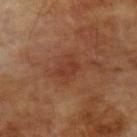{
  "biopsy_status": "not biopsied; imaged during a skin examination",
  "patient": {
    "sex": "male",
    "age_approx": 70
  },
  "site": "left upper arm",
  "image": {
    "source": "total-body photography crop",
    "field_of_view_mm": 15
  },
  "lighting": "cross-polarized",
  "lesion_size": {
    "long_diameter_mm_approx": 3.0
  }
}